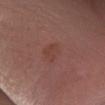The lesion was photographed on a routine skin check and not biopsied; there is no pathology result.